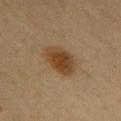• follow-up — total-body-photography surveillance lesion; no biopsy
• imaging modality — total-body-photography crop, ~15 mm field of view
• patient — female, aged 43–47
• lesion size — about 4.5 mm
• body site — the upper back